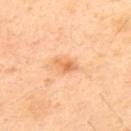Image and clinical context: On the upper back. Measured at roughly 3 mm in maximum diameter. This is a cross-polarized tile. An algorithmic analysis of the crop reported an average lesion color of about L≈70 a*≈25 b*≈43 (CIELAB), a lesion–skin lightness drop of about 10, and a normalized lesion–skin contrast near 6.5. It also reported peripheral color asymmetry of about 1.5. A 15 mm crop from a total-body photograph taken for skin-cancer surveillance. A male subject, about 50 years old.